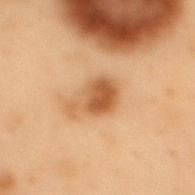{
  "biopsy_status": "not biopsied; imaged during a skin examination",
  "image": {
    "source": "total-body photography crop",
    "field_of_view_mm": 15
  },
  "patient": {
    "sex": "male",
    "age_approx": 55
  },
  "lesion_size": {
    "long_diameter_mm_approx": 4.5
  },
  "lighting": "cross-polarized",
  "site": "mid back",
  "automated_metrics": {
    "shape_asymmetry": 0.35
  }
}